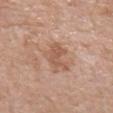No biopsy was performed on this lesion — it was imaged during a full skin examination and was not determined to be concerning.
A female patient aged approximately 70.
The lesion-visualizer software estimated an area of roughly 7.5 mm², an eccentricity of roughly 0.5, and a shape-asymmetry score of about 0.5 (0 = symmetric). The software also gave border irregularity of about 6 on a 0–10 scale and a peripheral color-asymmetry measure near 0.5. It also reported a classifier nevus-likeness of about 5/100 and lesion-presence confidence of about 100/100.
The lesion is located on the arm.
This image is a 15 mm lesion crop taken from a total-body photograph.